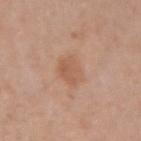Findings:
– lesion size · ≈3 mm
– tile lighting · white-light
– image · total-body-photography crop, ~15 mm field of view
– TBP lesion metrics · an average lesion color of about L≈57 a*≈21 b*≈32 (CIELAB), a lesion–skin lightness drop of about 7, and a normalized lesion–skin contrast near 5.5; border irregularity of about 2 on a 0–10 scale and a color-variation rating of about 2.5/10
– site · the arm
– subject · female, approximately 35 years of age